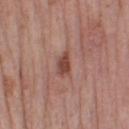{
  "site": "right thigh",
  "lighting": "white-light",
  "lesion_size": {
    "long_diameter_mm_approx": 2.5
  },
  "patient": {
    "sex": "female",
    "age_approx": 40
  },
  "image": {
    "source": "total-body photography crop",
    "field_of_view_mm": 15
  }
}